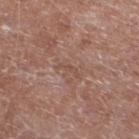<record>
<biopsy_status>not biopsied; imaged during a skin examination</biopsy_status>
<automated_metrics>
  <area_mm2_approx>2.0</area_mm2_approx>
  <eccentricity>0.95</eccentricity>
  <shape_asymmetry>0.7</shape_asymmetry>
  <cielab_L>49</cielab_L>
  <cielab_a>20</cielab_a>
  <cielab_b>26</cielab_b>
  <vs_skin_darker_L>6.0</vs_skin_darker_L>
  <vs_skin_contrast_norm>4.5</vs_skin_contrast_norm>
  <nevus_likeness_0_100>0</nevus_likeness_0_100>
  <lesion_detection_confidence_0_100>95</lesion_detection_confidence_0_100>
</automated_metrics>
<lesion_size>
  <long_diameter_mm_approx>3.0</long_diameter_mm_approx>
</lesion_size>
<image>
  <source>total-body photography crop</source>
  <field_of_view_mm>15</field_of_view_mm>
</image>
<lighting>white-light</lighting>
<patient>
  <sex>male</sex>
  <age_approx>60</age_approx>
</patient>
<site>leg</site>
</record>